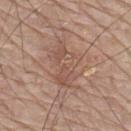<record>
  <biopsy_status>not biopsied; imaged during a skin examination</biopsy_status>
</record>